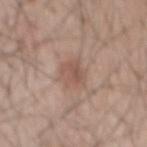No biopsy was performed on this lesion — it was imaged during a full skin examination and was not determined to be concerning. A roughly 15 mm field-of-view crop from a total-body skin photograph. On the mid back. The tile uses white-light illumination. The patient is a male aged around 50.